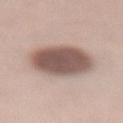Clinical impression: No biopsy was performed on this lesion — it was imaged during a full skin examination and was not determined to be concerning. Acquisition and patient details: The lesion-visualizer software estimated a lesion area of about 24 mm², a shape eccentricity near 0.55, and a symmetry-axis asymmetry near 0.05. It also reported a mean CIELAB color near L≈54 a*≈16 b*≈22, about 17 CIELAB-L* units darker than the surrounding skin, and a lesion-to-skin contrast of about 11 (normalized; higher = more distinct). The analysis additionally found peripheral color asymmetry of about 1. The analysis additionally found an automated nevus-likeness rating near 25 out of 100 and a detector confidence of about 100 out of 100 that the crop contains a lesion. Imaged with white-light lighting. Longest diameter approximately 6 mm. A 15 mm close-up extracted from a 3D total-body photography capture. On the back. A female patient, in their 40s.